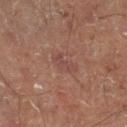Background: A male patient, roughly 50 years of age. This image is a 15 mm lesion crop taken from a total-body photograph. About 2.5 mm across. From the leg.